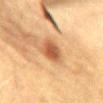Recorded during total-body skin imaging; not selected for excision or biopsy.
A female patient, in their 30s.
On the abdomen.
Longest diameter approximately 3 mm.
A close-up tile cropped from a whole-body skin photograph, about 15 mm across.
Imaged with cross-polarized lighting.
Automated image analysis of the tile measured a nevus-likeness score of about 95/100 and a lesion-detection confidence of about 100/100.